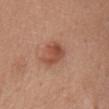biopsy status: catalogued during a skin exam; not biopsied
location: the chest
patient: female, aged 53–57
diameter: ~3.5 mm (longest diameter)
lighting: white-light
imaging modality: ~15 mm tile from a whole-body skin photo